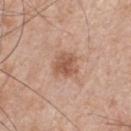The lesion was photographed on a routine skin check and not biopsied; there is no pathology result. A region of skin cropped from a whole-body photographic capture, roughly 15 mm wide. This is a white-light tile. The subject is a male aged around 50. Located on the chest.